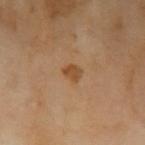Impression: Captured during whole-body skin photography for melanoma surveillance; the lesion was not biopsied. Context: On the left upper arm. Longest diameter approximately 2 mm. Automated image analysis of the tile measured a within-lesion color-variation index near 1.5/10 and a peripheral color-asymmetry measure near 0.5. A male subject aged around 70. A lesion tile, about 15 mm wide, cut from a 3D total-body photograph.